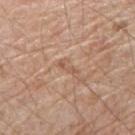Imaged during a routine full-body skin examination; the lesion was not biopsied and no histopathology is available. A male patient, about 75 years old. A close-up tile cropped from a whole-body skin photograph, about 15 mm across. Automated image analysis of the tile measured a classifier nevus-likeness of about 0/100 and lesion-presence confidence of about 75/100. The tile uses white-light illumination. On the right upper arm. The recorded lesion diameter is about 2.5 mm.